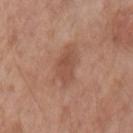Q: Is there a histopathology result?
A: total-body-photography surveillance lesion; no biopsy
Q: Patient demographics?
A: male, aged around 55
Q: Where on the body is the lesion?
A: the left upper arm
Q: What kind of image is this?
A: 15 mm crop, total-body photography